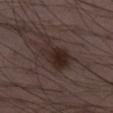• workup — no biopsy performed (imaged during a skin exam)
• acquisition — ~15 mm tile from a whole-body skin photo
• patient — male, aged 38–42
• TBP lesion metrics — a lesion area of about 10 mm², a shape eccentricity near 0.75, and two-axis asymmetry of about 0.3; a lesion color around L≈25 a*≈14 b*≈17 in CIELAB, roughly 9 lightness units darker than nearby skin, and a lesion-to-skin contrast of about 9.5 (normalized; higher = more distinct); a border-irregularity rating of about 3.5/10, a color-variation rating of about 4.5/10, and a peripheral color-asymmetry measure near 1.5
• anatomic site — the left lower leg